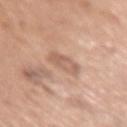• workup · imaged on a skin check; not biopsied
• location · the arm
• patient · female, aged 48–52
• acquisition · ~15 mm tile from a whole-body skin photo
• automated lesion analysis · a footprint of about 6.5 mm² and a symmetry-axis asymmetry near 0.25; a color-variation rating of about 3.5/10 and radial color variation of about 1; a classifier nevus-likeness of about 0/100 and lesion-presence confidence of about 100/100
• lesion diameter · about 3 mm
• lighting · white-light